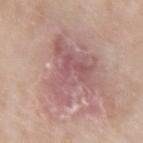biopsy status=imaged on a skin check; not biopsied